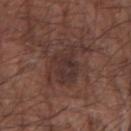This lesion was catalogued during total-body skin photography and was not selected for biopsy.
The total-body-photography lesion software estimated about 7 CIELAB-L* units darker than the surrounding skin and a normalized lesion–skin contrast near 6.5. It also reported a within-lesion color-variation index near 4/10. It also reported lesion-presence confidence of about 100/100.
The patient is a male roughly 65 years of age.
Cropped from a total-body skin-imaging series; the visible field is about 15 mm.
On the right forearm.
Imaged with white-light lighting.
About 5.5 mm across.